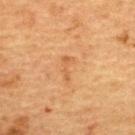Findings:
* biopsy status: total-body-photography surveillance lesion; no biopsy
* subject: female, aged 68–72
* body site: the upper back
* tile lighting: cross-polarized illumination
* imaging modality: total-body-photography crop, ~15 mm field of view